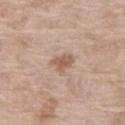<lesion>
  <biopsy_status>not biopsied; imaged during a skin examination</biopsy_status>
  <patient>
    <sex>female</sex>
    <age_approx>75</age_approx>
  </patient>
  <image>
    <source>total-body photography crop</source>
    <field_of_view_mm>15</field_of_view_mm>
  </image>
  <site>right thigh</site>
</lesion>